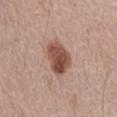The lesion was photographed on a routine skin check and not biopsied; there is no pathology result.
Automated tile analysis of the lesion measured a lesion–skin lightness drop of about 15 and a normalized border contrast of about 10.5. And it measured a border-irregularity index near 2/10, a within-lesion color-variation index near 6/10, and a peripheral color-asymmetry measure near 2.5.
A 15 mm crop from a total-body photograph taken for skin-cancer surveillance.
On the abdomen.
A male subject approximately 65 years of age.
Measured at roughly 4.5 mm in maximum diameter.